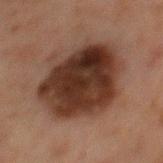biopsy status — imaged on a skin check; not biopsied | automated lesion analysis — a footprint of about 44 mm², an eccentricity of roughly 0.55, and a symmetry-axis asymmetry near 0.15; a border-irregularity rating of about 2/10, a color-variation rating of about 6/10, and radial color variation of about 2 | patient — male, about 65 years old | location — the abdomen | lesion size — ≈8 mm | acquisition — ~15 mm tile from a whole-body skin photo | illumination — cross-polarized.